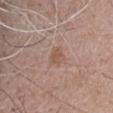notes=total-body-photography surveillance lesion; no biopsy
tile lighting=white-light illumination
lesion size=~2.5 mm (longest diameter)
anatomic site=the head or neck
imaging modality=total-body-photography crop, ~15 mm field of view
TBP lesion metrics=border irregularity of about 2.5 on a 0–10 scale, a within-lesion color-variation index near 2/10, and peripheral color asymmetry of about 1; an automated nevus-likeness rating near 0 out of 100
subject=male, aged 68 to 72